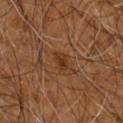biopsy status — imaged on a skin check; not biopsied